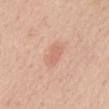Captured during whole-body skin photography for melanoma surveillance; the lesion was not biopsied.